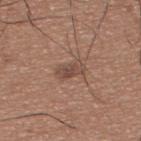Q: Is there a histopathology result?
A: catalogued during a skin exam; not biopsied
Q: How large is the lesion?
A: about 3 mm
Q: What did automated image analysis measure?
A: a shape eccentricity near 0.85 and a shape-asymmetry score of about 0.2 (0 = symmetric); a lesion–skin lightness drop of about 9 and a lesion-to-skin contrast of about 6.5 (normalized; higher = more distinct); a classifier nevus-likeness of about 20/100 and a detector confidence of about 100 out of 100 that the crop contains a lesion
Q: What is the imaging modality?
A: 15 mm crop, total-body photography
Q: Where on the body is the lesion?
A: the upper back
Q: Who is the patient?
A: male, aged around 35